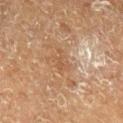The lesion was photographed on a routine skin check and not biopsied; there is no pathology result. The lesion-visualizer software estimated a footprint of about 3 mm², a shape eccentricity near 0.85, and a symmetry-axis asymmetry near 0.65. The software also gave a lesion color around L≈42 a*≈17 b*≈28 in CIELAB, a lesion–skin lightness drop of about 5, and a normalized lesion–skin contrast near 5. It also reported a nevus-likeness score of about 0/100. On the right lower leg. A lesion tile, about 15 mm wide, cut from a 3D total-body photograph. Measured at roughly 2.5 mm in maximum diameter. A male subject in their mid-70s. Captured under cross-polarized illumination.